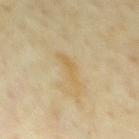Findings:
• notes: total-body-photography surveillance lesion; no biopsy
• image source: 15 mm crop, total-body photography
• patient: male, aged 63–67
• location: the chest
• lesion size: about 4 mm
• tile lighting: cross-polarized illumination